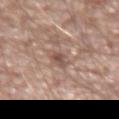Assessment: Imaged during a routine full-body skin examination; the lesion was not biopsied and no histopathology is available. Acquisition and patient details: A close-up tile cropped from a whole-body skin photograph, about 15 mm across. A male patient, roughly 75 years of age. Captured under white-light illumination. About 2.5 mm across. On the right forearm.